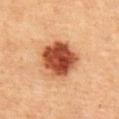Findings:
- biopsy status · catalogued during a skin exam; not biopsied
- location · the chest
- image · 15 mm crop, total-body photography
- patient · female, aged approximately 60
- size · about 5 mm
- lighting · cross-polarized
- automated lesion analysis · a border-irregularity rating of about 1.5/10 and a color-variation rating of about 6/10; a detector confidence of about 100 out of 100 that the crop contains a lesion